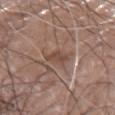Q: Is there a histopathology result?
A: no biopsy performed (imaged during a skin exam)
Q: Who is the patient?
A: male, aged 78–82
Q: How was this image acquired?
A: 15 mm crop, total-body photography
Q: How was the tile lit?
A: white-light
Q: Lesion location?
A: the left arm
Q: Automated lesion metrics?
A: an area of roughly 4 mm² and a symmetry-axis asymmetry near 0.25; a within-lesion color-variation index near 1.5/10 and peripheral color asymmetry of about 0.5